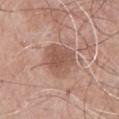| field | value |
|---|---|
| notes | total-body-photography surveillance lesion; no biopsy |
| automated lesion analysis | a lesion color around L≈54 a*≈20 b*≈27 in CIELAB; peripheral color asymmetry of about 1 |
| image | 15 mm crop, total-body photography |
| body site | the chest |
| patient | male, aged around 65 |
| illumination | white-light illumination |